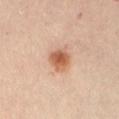* follow-up: catalogued during a skin exam; not biopsied
* anatomic site: the front of the torso
* image source: ~15 mm crop, total-body skin-cancer survey
* diameter: about 3 mm
* patient: female, roughly 65 years of age
* image-analysis metrics: peripheral color asymmetry of about 1; an automated nevus-likeness rating near 100 out of 100 and a detector confidence of about 100 out of 100 that the crop contains a lesion